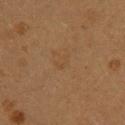  patient:
    sex: female
    age_approx: 20
  image:
    source: total-body photography crop
    field_of_view_mm: 15
  site: upper back
  automated_metrics:
    area_mm2_approx: 2.5
    eccentricity: 0.7
    shape_asymmetry: 0.3
    lesion_detection_confidence_0_100: 100
  lesion_size:
    long_diameter_mm_approx: 2.0
  lighting: cross-polarized